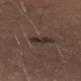workup=no biopsy performed (imaged during a skin exam) | body site=the right thigh | image source=~15 mm crop, total-body skin-cancer survey | subject=female, about 30 years old | lesion diameter=~3 mm (longest diameter) | lighting=white-light.